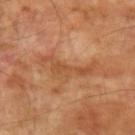Notes:
– follow-up: no biopsy performed (imaged during a skin exam)
– diameter: ≈6 mm
– site: the left upper arm
– TBP lesion metrics: a footprint of about 8 mm², a shape eccentricity near 0.95, and a shape-asymmetry score of about 0.6 (0 = symmetric); a nevus-likeness score of about 0/100
– image source: ~15 mm tile from a whole-body skin photo
– subject: male, aged 68 to 72
– illumination: cross-polarized